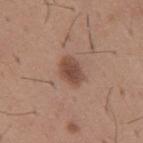Case summary:
* acquisition — total-body-photography crop, ~15 mm field of view
* anatomic site — the mid back
* image-analysis metrics — an area of roughly 6 mm² and an outline eccentricity of about 0.75 (0 = round, 1 = elongated); a border-irregularity index near 1.5/10 and a color-variation rating of about 2.5/10; a nevus-likeness score of about 95/100 and a detector confidence of about 100 out of 100 that the crop contains a lesion
* subject — male, about 65 years old
* size — ≈3.5 mm